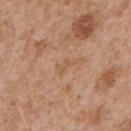The lesion was photographed on a routine skin check and not biopsied; there is no pathology result.